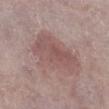Q: Was this lesion biopsied?
A: total-body-photography surveillance lesion; no biopsy
Q: Lesion location?
A: the right lower leg
Q: What are the patient's age and sex?
A: male, aged around 80
Q: How was the tile lit?
A: white-light
Q: How was this image acquired?
A: 15 mm crop, total-body photography